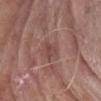notes: no biopsy performed (imaged during a skin exam) | automated metrics: a footprint of about 3.5 mm², an outline eccentricity of about 0.8 (0 = round, 1 = elongated), and two-axis asymmetry of about 0.35 | subject: male, aged approximately 75 | body site: the right forearm | diameter: about 2.5 mm | image source: ~15 mm crop, total-body skin-cancer survey | tile lighting: white-light.